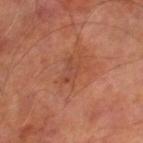Impression: Captured during whole-body skin photography for melanoma surveillance; the lesion was not biopsied. Clinical summary: The lesion's longest dimension is about 3 mm. An algorithmic analysis of the crop reported an area of roughly 4.5 mm², an outline eccentricity of about 0.8 (0 = round, 1 = elongated), and two-axis asymmetry of about 0.4. And it measured an average lesion color of about L≈45 a*≈25 b*≈30 (CIELAB), about 6 CIELAB-L* units darker than the surrounding skin, and a normalized lesion–skin contrast near 4.5. The software also gave a border-irregularity rating of about 4.5/10 and a color-variation rating of about 2/10. The lesion is on the right lower leg. Imaged with cross-polarized lighting. Cropped from a total-body skin-imaging series; the visible field is about 15 mm. The subject is a male aged approximately 70.